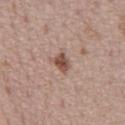<lesion>
<lighting>white-light</lighting>
<image>
  <source>total-body photography crop</source>
  <field_of_view_mm>15</field_of_view_mm>
</image>
<automated_metrics>
  <area_mm2_approx>4.0</area_mm2_approx>
  <shape_asymmetry>0.3</shape_asymmetry>
  <border_irregularity_0_10>2.5</border_irregularity_0_10>
  <color_variation_0_10>3.0</color_variation_0_10>
  <peripheral_color_asymmetry>1.0</peripheral_color_asymmetry>
  <nevus_likeness_0_100>85</nevus_likeness_0_100>
  <lesion_detection_confidence_0_100>100</lesion_detection_confidence_0_100>
</automated_metrics>
<patient>
  <sex>male</sex>
  <age_approx>75</age_approx>
</patient>
<lesion_size>
  <long_diameter_mm_approx>2.5</long_diameter_mm_approx>
</lesion_size>
<site>chest</site>
</lesion>